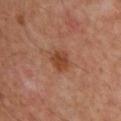  biopsy_status: not biopsied; imaged during a skin examination
  lighting: cross-polarized
  image:
    source: total-body photography crop
    field_of_view_mm: 15
  patient:
    sex: male
    age_approx: 50
  site: arm
  lesion_size:
    long_diameter_mm_approx: 2.5